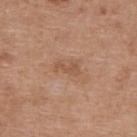| key | value |
|---|---|
| lesion diameter | ~3 mm (longest diameter) |
| anatomic site | the upper back |
| image | total-body-photography crop, ~15 mm field of view |
| subject | female, aged around 40 |